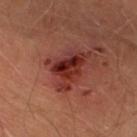<tbp_lesion>
<biopsy_status>not biopsied; imaged during a skin examination</biopsy_status>
<site>left lower leg</site>
<automated_metrics>
  <cielab_L>33</cielab_L>
  <cielab_a>30</cielab_a>
  <cielab_b>27</cielab_b>
  <vs_skin_contrast_norm>10.5</vs_skin_contrast_norm>
  <border_irregularity_0_10>6.0</border_irregularity_0_10>
  <color_variation_0_10>8.5</color_variation_0_10>
</automated_metrics>
<lesion_size>
  <long_diameter_mm_approx>5.5</long_diameter_mm_approx>
</lesion_size>
<image>
  <source>total-body photography crop</source>
  <field_of_view_mm>15</field_of_view_mm>
</image>
<patient>
  <sex>male</sex>
  <age_approx>40</age_approx>
</patient>
<lighting>cross-polarized</lighting>
</tbp_lesion>